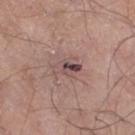{"biopsy_status": "not biopsied; imaged during a skin examination", "automated_metrics": {"cielab_L": 48, "cielab_a": 18, "cielab_b": 19, "vs_skin_darker_L": 10.0, "vs_skin_contrast_norm": 8.0, "border_irregularity_0_10": 3.5, "color_variation_0_10": 9.0, "peripheral_color_asymmetry": 5.0, "nevus_likeness_0_100": 0}, "site": "right thigh", "image": {"source": "total-body photography crop", "field_of_view_mm": 15}, "patient": {"sex": "male", "age_approx": 70}}